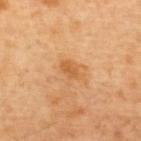<case>
<biopsy_status>not biopsied; imaged during a skin examination</biopsy_status>
<patient>
  <sex>male</sex>
  <age_approx>60</age_approx>
</patient>
<site>upper back</site>
<image>
  <source>total-body photography crop</source>
  <field_of_view_mm>15</field_of_view_mm>
</image>
</case>